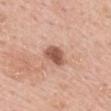follow-up: no biopsy performed (imaged during a skin exam)
image source: ~15 mm crop, total-body skin-cancer survey
body site: the mid back
patient: male, approximately 55 years of age
diameter: ~3 mm (longest diameter)
lighting: white-light illumination
image-analysis metrics: a footprint of about 6 mm², a shape eccentricity near 0.6, and a shape-asymmetry score of about 0.2 (0 = symmetric); border irregularity of about 2 on a 0–10 scale, internal color variation of about 3 on a 0–10 scale, and a peripheral color-asymmetry measure near 1; a classifier nevus-likeness of about 90/100 and a detector confidence of about 100 out of 100 that the crop contains a lesion